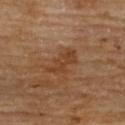  biopsy_status: not biopsied; imaged during a skin examination
  site: upper back
  image:
    source: total-body photography crop
    field_of_view_mm: 15
  patient:
    sex: male
    age_approx: 85+
  lighting: cross-polarized
  lesion_size:
    long_diameter_mm_approx: 4.5
  automated_metrics:
    cielab_L: 39
    cielab_a: 21
    cielab_b: 32
    vs_skin_contrast_norm: 6.5
    lesion_detection_confidence_0_100: 100